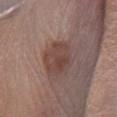workup: total-body-photography surveillance lesion; no biopsy | acquisition: ~15 mm tile from a whole-body skin photo | lesion size: ≈4 mm | patient: male, aged around 55 | anatomic site: the leg.